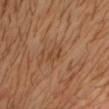This lesion was catalogued during total-body skin photography and was not selected for biopsy.
About 2.5 mm across.
Cropped from a total-body skin-imaging series; the visible field is about 15 mm.
A male patient in their 30s.
On the chest.